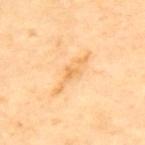{"biopsy_status": "not biopsied; imaged during a skin examination", "image": {"source": "total-body photography crop", "field_of_view_mm": 15}, "lighting": "cross-polarized", "automated_metrics": {"cielab_L": 76, "cielab_a": 21, "cielab_b": 47, "vs_skin_darker_L": 8.0, "vs_skin_contrast_norm": 5.5}, "patient": {"sex": "male", "age_approx": 65}, "site": "upper back"}